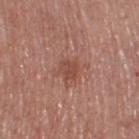<case>
<biopsy_status>not biopsied; imaged during a skin examination</biopsy_status>
<lighting>white-light</lighting>
<image>
  <source>total-body photography crop</source>
  <field_of_view_mm>15</field_of_view_mm>
</image>
<automated_metrics>
  <cielab_L>47</cielab_L>
  <cielab_a>24</cielab_a>
  <cielab_b>27</cielab_b>
  <vs_skin_darker_L>8.0</vs_skin_darker_L>
  <vs_skin_contrast_norm>6.0</vs_skin_contrast_norm>
  <color_variation_0_10>1.5</color_variation_0_10>
  <peripheral_color_asymmetry>0.5</peripheral_color_asymmetry>
  <nevus_likeness_0_100>0</nevus_likeness_0_100>
  <lesion_detection_confidence_0_100>100</lesion_detection_confidence_0_100>
</automated_metrics>
<site>right thigh</site>
<patient>
  <sex>male</sex>
  <age_approx>70</age_approx>
</patient>
<lesion_size>
  <long_diameter_mm_approx>2.5</long_diameter_mm_approx>
</lesion_size>
</case>